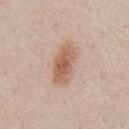* follow-up: no biopsy performed (imaged during a skin exam)
* anatomic site: the chest
* imaging modality: ~15 mm tile from a whole-body skin photo
* tile lighting: white-light illumination
* image-analysis metrics: a lesion color around L≈60 a*≈20 b*≈29 in CIELAB and a normalized border contrast of about 8; border irregularity of about 2.5 on a 0–10 scale, a within-lesion color-variation index near 4.5/10, and radial color variation of about 1.5; a classifier nevus-likeness of about 90/100 and lesion-presence confidence of about 100/100
* lesion size: ~5 mm (longest diameter)
* patient: male, in their 50s